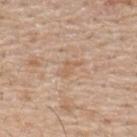The lesion is on the upper back. A close-up tile cropped from a whole-body skin photograph, about 15 mm across. A male patient, about 55 years old.